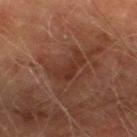| field | value |
|---|---|
| biopsy status | catalogued during a skin exam; not biopsied |
| lesion size | ≈4 mm |
| image-analysis metrics | an average lesion color of about L≈27 a*≈19 b*≈23 (CIELAB), roughly 6 lightness units darker than nearby skin, and a normalized lesion–skin contrast near 6.5; a border-irregularity index near 5.5/10, a color-variation rating of about 1.5/10, and a peripheral color-asymmetry measure near 0.5; a detector confidence of about 95 out of 100 that the crop contains a lesion |
| location | the right thigh |
| image | ~15 mm crop, total-body skin-cancer survey |
| patient | male, in their mid- to late 70s |
| tile lighting | cross-polarized |